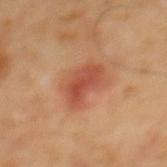This lesion was catalogued during total-body skin photography and was not selected for biopsy.
A 15 mm close-up extracted from a 3D total-body photography capture.
The lesion is located on the back.
Imaged with cross-polarized lighting.
The lesion's longest dimension is about 5 mm.
The patient is a male in their mid- to late 60s.